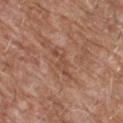Assessment: Imaged during a routine full-body skin examination; the lesion was not biopsied and no histopathology is available. Image and clinical context: An algorithmic analysis of the crop reported an eccentricity of roughly 0.9 and two-axis asymmetry of about 0.45. It also reported border irregularity of about 6 on a 0–10 scale and peripheral color asymmetry of about 0. And it measured a nevus-likeness score of about 0/100 and a detector confidence of about 70 out of 100 that the crop contains a lesion. On the chest. The subject is a male about 60 years old. About 4 mm across. Cropped from a total-body skin-imaging series; the visible field is about 15 mm.